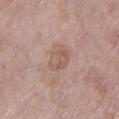Impression: Recorded during total-body skin imaging; not selected for excision or biopsy. Context: A close-up tile cropped from a whole-body skin photograph, about 15 mm across. Located on the left lower leg. The subject is a female aged 73 to 77. Longest diameter approximately 3 mm. Captured under white-light illumination.